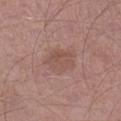Impression:
Part of a total-body skin-imaging series; this lesion was reviewed on a skin check and was not flagged for biopsy.
Background:
Located on the right lower leg. A male patient approximately 55 years of age. A roughly 15 mm field-of-view crop from a total-body skin photograph.